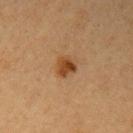Impression: Imaged during a routine full-body skin examination; the lesion was not biopsied and no histopathology is available. Context: Captured under cross-polarized illumination. A female subject roughly 40 years of age. A 15 mm close-up tile from a total-body photography series done for melanoma screening. Longest diameter approximately 2.5 mm. On the left upper arm.